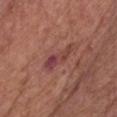Impression: Imaged during a routine full-body skin examination; the lesion was not biopsied and no histopathology is available. Clinical summary: A male patient, about 60 years old. A region of skin cropped from a whole-body photographic capture, roughly 15 mm wide. On the chest.